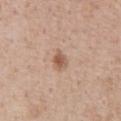<case>
  <biopsy_status>not biopsied; imaged during a skin examination</biopsy_status>
  <lesion_size>
    <long_diameter_mm_approx>3.0</long_diameter_mm_approx>
  </lesion_size>
  <image>
    <source>total-body photography crop</source>
    <field_of_view_mm>15</field_of_view_mm>
  </image>
  <patient>
    <sex>male</sex>
    <age_approx>55</age_approx>
  </patient>
  <site>front of the torso</site>
</case>